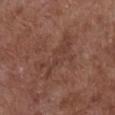biopsy_status: not biopsied; imaged during a skin examination
automated_metrics:
  border_irregularity_0_10: 8.5
  color_variation_0_10: 1.5
  peripheral_color_asymmetry: 0.5
  nevus_likeness_0_100: 0
  lesion_detection_confidence_0_100: 90
patient:
  sex: female
  age_approx: 80
lighting: white-light
image:
  source: total-body photography crop
  field_of_view_mm: 15
lesion_size:
  long_diameter_mm_approx: 6.5
site: chest